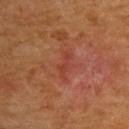Case summary:
• automated lesion analysis · a lesion area of about 2.5 mm², a shape eccentricity near 0.95, and two-axis asymmetry of about 0.55; a lesion–skin lightness drop of about 5 and a normalized border contrast of about 5; border irregularity of about 7 on a 0–10 scale, a within-lesion color-variation index near 0/10, and radial color variation of about 0
• lighting · cross-polarized illumination
• imaging modality · 15 mm crop, total-body photography
• body site · the upper back
• patient · male, aged 63 to 67